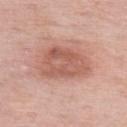Captured during whole-body skin photography for melanoma surveillance; the lesion was not biopsied. The lesion is located on the upper back. A female subject, roughly 50 years of age. A lesion tile, about 15 mm wide, cut from a 3D total-body photograph. Measured at roughly 6.5 mm in maximum diameter. This is a white-light tile.